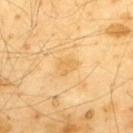The lesion was tiled from a total-body skin photograph and was not biopsied.
Measured at roughly 2.5 mm in maximum diameter.
On the upper back.
Cropped from a total-body skin-imaging series; the visible field is about 15 mm.
The lesion-visualizer software estimated a lesion area of about 4 mm², an eccentricity of roughly 0.6, and a shape-asymmetry score of about 0.35 (0 = symmetric). And it measured a mean CIELAB color near L≈73 a*≈19 b*≈50, about 6 CIELAB-L* units darker than the surrounding skin, and a lesion-to-skin contrast of about 5 (normalized; higher = more distinct). The analysis additionally found a border-irregularity rating of about 3.5/10 and radial color variation of about 0.5. And it measured a classifier nevus-likeness of about 0/100 and lesion-presence confidence of about 100/100.
The patient is a male in their mid- to late 60s.
Imaged with cross-polarized lighting.